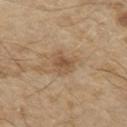The lesion was tiled from a total-body skin photograph and was not biopsied.
Located on the chest.
A 15 mm close-up extracted from a 3D total-body photography capture.
A male patient, aged 68 to 72.